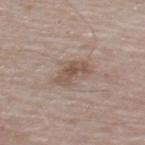This lesion was catalogued during total-body skin photography and was not selected for biopsy.
On the back.
The tile uses white-light illumination.
About 3.5 mm across.
A male subject, aged 73–77.
Cropped from a total-body skin-imaging series; the visible field is about 15 mm.